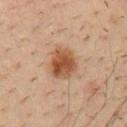site = the chest | lesion diameter = about 4 mm | subject = male, aged around 35 | image source = ~15 mm crop, total-body skin-cancer survey | illumination = cross-polarized illumination.